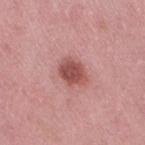Assessment:
No biopsy was performed on this lesion — it was imaged during a full skin examination and was not determined to be concerning.
Clinical summary:
About 3.5 mm across. A 15 mm close-up tile from a total-body photography series done for melanoma screening. The tile uses white-light illumination. A female patient aged 38 to 42. The lesion is on the leg.